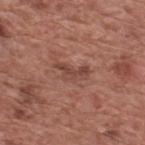Imaged during a routine full-body skin examination; the lesion was not biopsied and no histopathology is available. From the back. A roughly 15 mm field-of-view crop from a total-body skin photograph. Longest diameter approximately 4 mm. The tile uses white-light illumination. Automated tile analysis of the lesion measured about 8 CIELAB-L* units darker than the surrounding skin and a normalized lesion–skin contrast near 6.5. The analysis additionally found a border-irregularity index near 6.5/10, a within-lesion color-variation index near 2.5/10, and peripheral color asymmetry of about 0.5. A male subject, in their mid-70s.